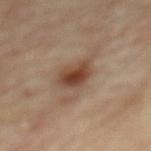The tile uses cross-polarized illumination. The recorded lesion diameter is about 3 mm. A male subject about 85 years old or older. A 15 mm close-up tile from a total-body photography series done for melanoma screening. Located on the mid back.